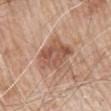Recorded during total-body skin imaging; not selected for excision or biopsy. From the mid back. About 5.5 mm across. Imaged with white-light lighting. A close-up tile cropped from a whole-body skin photograph, about 15 mm across. The patient is a male aged around 80.